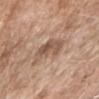Assessment:
Imaged during a routine full-body skin examination; the lesion was not biopsied and no histopathology is available.
Background:
Measured at roughly 4 mm in maximum diameter. A 15 mm close-up tile from a total-body photography series done for melanoma screening. A female subject, approximately 75 years of age. Imaged with white-light lighting. The lesion is on the right upper arm.